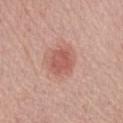Findings:
• follow-up: no biopsy performed (imaged during a skin exam)
• image: total-body-photography crop, ~15 mm field of view
• anatomic site: the left forearm
• patient: female, aged 63–67